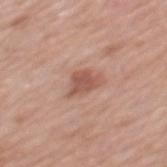The lesion is located on the back. The lesion-visualizer software estimated a lesion area of about 5.5 mm², an outline eccentricity of about 0.7 (0 = round, 1 = elongated), and a shape-asymmetry score of about 0.35 (0 = symmetric). It also reported a within-lesion color-variation index near 2/10 and radial color variation of about 0.5. The subject is a female aged around 60. Approximately 3.5 mm at its widest. Imaged with white-light lighting. This image is a 15 mm lesion crop taken from a total-body photograph.